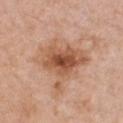follow-up: no biopsy performed (imaged during a skin exam).